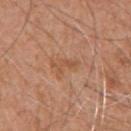Part of a total-body skin-imaging series; this lesion was reviewed on a skin check and was not flagged for biopsy. From the front of the torso. A male patient about 55 years old. The recorded lesion diameter is about 3.5 mm. A lesion tile, about 15 mm wide, cut from a 3D total-body photograph. Imaged with white-light lighting.